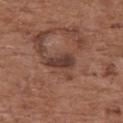{"biopsy_status": "not biopsied; imaged during a skin examination", "lighting": "white-light", "automated_metrics": {"cielab_L": 38, "cielab_a": 20, "cielab_b": 23, "vs_skin_contrast_norm": 9.0, "nevus_likeness_0_100": 5}, "image": {"source": "total-body photography crop", "field_of_view_mm": 15}, "patient": {"sex": "female", "age_approx": 75}, "site": "upper back", "lesion_size": {"long_diameter_mm_approx": 3.5}}